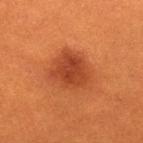  biopsy_status: not biopsied; imaged during a skin examination
  site: right lower leg
  lesion_size:
    long_diameter_mm_approx: 5.0
  patient:
    sex: female
    age_approx: 30
  image:
    source: total-body photography crop
    field_of_view_mm: 15
  lighting: cross-polarized